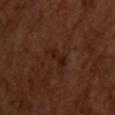Part of a total-body skin-imaging series; this lesion was reviewed on a skin check and was not flagged for biopsy. Automated image analysis of the tile measured a lesion area of about 3.5 mm², an outline eccentricity of about 0.9 (0 = round, 1 = elongated), and a symmetry-axis asymmetry near 0.45. And it measured a mean CIELAB color near L≈20 a*≈20 b*≈24, roughly 6 lightness units darker than nearby skin, and a normalized border contrast of about 7. Approximately 3.5 mm at its widest. A roughly 15 mm field-of-view crop from a total-body skin photograph. A male patient, in their 50s. Located on the upper back. The tile uses cross-polarized illumination.